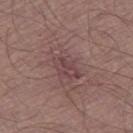Clinical impression:
The lesion was tiled from a total-body skin photograph and was not biopsied.
Background:
The lesion is on the right thigh. A 15 mm close-up extracted from a 3D total-body photography capture. The subject is a male in their mid-50s.